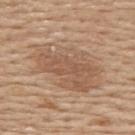<tbp_lesion>
  <biopsy_status>not biopsied; imaged during a skin examination</biopsy_status>
  <patient>
    <sex>female</sex>
    <age_approx>60</age_approx>
  </patient>
  <image>
    <source>total-body photography crop</source>
    <field_of_view_mm>15</field_of_view_mm>
  </image>
  <site>upper back</site>
</tbp_lesion>